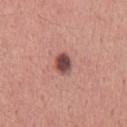workup: catalogued during a skin exam; not biopsied | patient: male, approximately 45 years of age | image-analysis metrics: border irregularity of about 1.5 on a 0–10 scale, a color-variation rating of about 2.5/10, and a peripheral color-asymmetry measure near 0.5; a classifier nevus-likeness of about 95/100 and a detector confidence of about 100 out of 100 that the crop contains a lesion | site: the chest | lesion diameter: ≈2.5 mm | imaging modality: ~15 mm tile from a whole-body skin photo | lighting: white-light illumination.